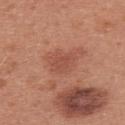Assessment:
The lesion was photographed on a routine skin check and not biopsied; there is no pathology result.
Acquisition and patient details:
The lesion is located on the upper back. A region of skin cropped from a whole-body photographic capture, roughly 15 mm wide. The patient is a female roughly 40 years of age. The recorded lesion diameter is about 3 mm. This is a white-light tile. Automated image analysis of the tile measured an average lesion color of about L≈50 a*≈27 b*≈30 (CIELAB) and a lesion–skin lightness drop of about 7. And it measured a border-irregularity index near 3/10, a color-variation rating of about 1.5/10, and radial color variation of about 0.5. It also reported lesion-presence confidence of about 100/100.